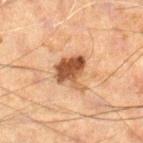The lesion was tiled from a total-body skin photograph and was not biopsied. Located on the leg. A close-up tile cropped from a whole-body skin photograph, about 15 mm across. The tile uses cross-polarized illumination. The subject is a male roughly 60 years of age.